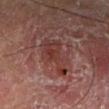A male patient, aged 53 to 57. On the leg. Longest diameter approximately 5.5 mm. An algorithmic analysis of the crop reported a nevus-likeness score of about 0/100 and a detector confidence of about 90 out of 100 that the crop contains a lesion. A 15 mm crop from a total-body photograph taken for skin-cancer surveillance. The tile uses cross-polarized illumination.